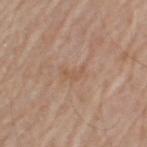Imaged during a routine full-body skin examination; the lesion was not biopsied and no histopathology is available. A male patient aged 78–82. The lesion's longest dimension is about 2.5 mm. A close-up tile cropped from a whole-body skin photograph, about 15 mm across. The lesion is located on the left upper arm. The tile uses white-light illumination.